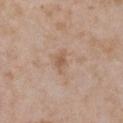The tile uses white-light illumination. The lesion's longest dimension is about 3 mm. A female subject aged approximately 30. This image is a 15 mm lesion crop taken from a total-body photograph. From the chest.